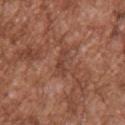| key | value |
|---|---|
| workup | no biopsy performed (imaged during a skin exam) |
| automated lesion analysis | border irregularity of about 4.5 on a 0–10 scale, a color-variation rating of about 0/10, and a peripheral color-asymmetry measure near 0; a detector confidence of about 80 out of 100 that the crop contains a lesion |
| location | the upper back |
| image source | ~15 mm crop, total-body skin-cancer survey |
| lighting | white-light illumination |
| patient | male, approximately 45 years of age |